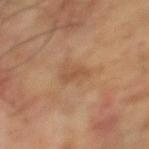subject=male, roughly 65 years of age
site=the left lower leg
acquisition=15 mm crop, total-body photography